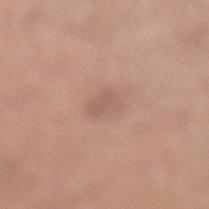image — ~15 mm tile from a whole-body skin photo | TBP lesion metrics — an area of roughly 4.5 mm², an outline eccentricity of about 0.7 (0 = round, 1 = elongated), and a symmetry-axis asymmetry near 0.25; a lesion color around L≈58 a*≈19 b*≈25 in CIELAB and about 7 CIELAB-L* units darker than the surrounding skin; a border-irregularity rating of about 2/10 and peripheral color asymmetry of about 0.5; a classifier nevus-likeness of about 0/100 | patient — male, in their 40s | lighting — white-light illumination | size — ≈2.5 mm | site — the left lower leg.